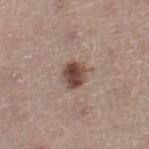Clinical impression:
Recorded during total-body skin imaging; not selected for excision or biopsy.
Image and clinical context:
The lesion's longest dimension is about 3 mm. A female subject, aged approximately 50. The lesion is located on the right lower leg. This is a white-light tile. A lesion tile, about 15 mm wide, cut from a 3D total-body photograph. The total-body-photography lesion software estimated a footprint of about 6.5 mm², an outline eccentricity of about 0.55 (0 = round, 1 = elongated), and a shape-asymmetry score of about 0.2 (0 = symmetric). The software also gave a lesion color around L≈46 a*≈17 b*≈24 in CIELAB and a normalized lesion–skin contrast near 10.5. The software also gave an automated nevus-likeness rating near 95 out of 100 and lesion-presence confidence of about 100/100.